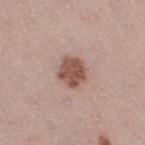Captured during whole-body skin photography for melanoma surveillance; the lesion was not biopsied. A 15 mm close-up tile from a total-body photography series done for melanoma screening. About 3.5 mm across. A female patient, aged approximately 40. From the leg. Automated image analysis of the tile measured a shape eccentricity near 0.5 and a shape-asymmetry score of about 0.2 (0 = symmetric). The software also gave a classifier nevus-likeness of about 85/100 and lesion-presence confidence of about 100/100. The tile uses white-light illumination.